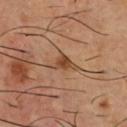The lesion was tiled from a total-body skin photograph and was not biopsied.